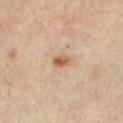{
  "biopsy_status": "not biopsied; imaged during a skin examination",
  "automated_metrics": {
    "eccentricity": 0.75,
    "shape_asymmetry": 0.2
  },
  "site": "left upper arm",
  "lighting": "cross-polarized",
  "patient": {
    "sex": "male",
    "age_approx": 45
  },
  "lesion_size": {
    "long_diameter_mm_approx": 2.5
  },
  "image": {
    "source": "total-body photography crop",
    "field_of_view_mm": 15
  }
}